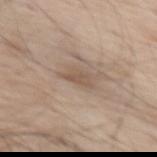Recorded during total-body skin imaging; not selected for excision or biopsy. On the front of the torso. An algorithmic analysis of the crop reported an average lesion color of about L≈54 a*≈15 b*≈27 (CIELAB), a lesion–skin lightness drop of about 8, and a lesion-to-skin contrast of about 6 (normalized; higher = more distinct). It also reported a classifier nevus-likeness of about 5/100 and a detector confidence of about 95 out of 100 that the crop contains a lesion. This is a white-light tile. A roughly 15 mm field-of-view crop from a total-body skin photograph. A male subject, roughly 70 years of age.